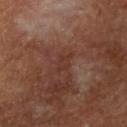The lesion was tiled from a total-body skin photograph and was not biopsied. A 15 mm close-up extracted from a 3D total-body photography capture. Located on the arm. The subject is a male roughly 65 years of age.